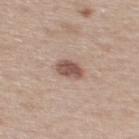Clinical impression:
Part of a total-body skin-imaging series; this lesion was reviewed on a skin check and was not flagged for biopsy.
Clinical summary:
Located on the upper back. Imaged with white-light lighting. A female subject aged 38 to 42. This image is a 15 mm lesion crop taken from a total-body photograph.